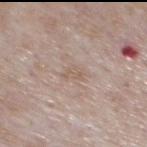This is a white-light tile.
Automated tile analysis of the lesion measured a lesion color around L≈59 a*≈15 b*≈25 in CIELAB, roughly 6 lightness units darker than nearby skin, and a normalized border contrast of about 4.5. And it measured internal color variation of about 1.5 on a 0–10 scale and peripheral color asymmetry of about 0.5.
A male subject approximately 75 years of age.
The recorded lesion diameter is about 2.5 mm.
From the mid back.
A 15 mm crop from a total-body photograph taken for skin-cancer surveillance.